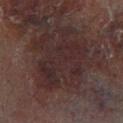Captured during whole-body skin photography for melanoma surveillance; the lesion was not biopsied. The lesion is on the right lower leg. A male patient approximately 60 years of age. A 15 mm close-up extracted from a 3D total-body photography capture.